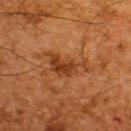No biopsy was performed on this lesion — it was imaged during a full skin examination and was not determined to be concerning. Approximately 6 mm at its widest. This is a cross-polarized tile. A region of skin cropped from a whole-body photographic capture, roughly 15 mm wide. On the upper back. The lesion-visualizer software estimated a lesion-detection confidence of about 100/100. The subject is a male roughly 65 years of age.